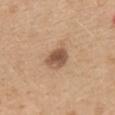follow-up: total-body-photography surveillance lesion; no biopsy
subject: female, aged around 45
site: the mid back
image: ~15 mm crop, total-body skin-cancer survey
automated metrics: a lesion color around L≈52 a*≈19 b*≈30 in CIELAB, a lesion–skin lightness drop of about 14, and a normalized border contrast of about 9.5
lesion diameter: about 3 mm